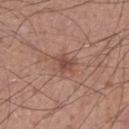Imaged during a routine full-body skin examination; the lesion was not biopsied and no histopathology is available. A close-up tile cropped from a whole-body skin photograph, about 15 mm across. A male subject, in their 30s. Approximately 3 mm at its widest. The lesion is on the left lower leg. This is a white-light tile.